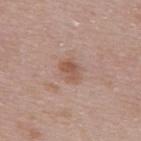Captured under white-light illumination.
Cropped from a whole-body photographic skin survey; the tile spans about 15 mm.
On the upper back.
A female patient, aged approximately 30.